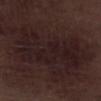Clinical impression:
Captured during whole-body skin photography for melanoma surveillance; the lesion was not biopsied.
Acquisition and patient details:
Automated image analysis of the tile measured a lesion area of about 38 mm², an outline eccentricity of about 0.85 (0 = round, 1 = elongated), and a symmetry-axis asymmetry near 0.25. The software also gave an average lesion color of about L≈18 a*≈14 b*≈13 (CIELAB) and a lesion–skin lightness drop of about 5. It also reported border irregularity of about 6 on a 0–10 scale and a within-lesion color-variation index near 3/10. The analysis additionally found an automated nevus-likeness rating near 0 out of 100 and a lesion-detection confidence of about 95/100. A roughly 15 mm field-of-view crop from a total-body skin photograph. From the left lower leg. The lesion's longest dimension is about 9.5 mm. The tile uses white-light illumination. A male patient, aged approximately 70.